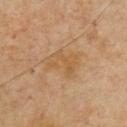Notes:
• follow-up — total-body-photography surveillance lesion; no biopsy
• illumination — cross-polarized illumination
• anatomic site — the chest
• automated lesion analysis — an area of roughly 8.5 mm², a shape eccentricity near 0.7, and a shape-asymmetry score of about 0.35 (0 = symmetric); a mean CIELAB color near L≈44 a*≈15 b*≈31 and a lesion–skin lightness drop of about 5; internal color variation of about 2 on a 0–10 scale and peripheral color asymmetry of about 1; a nevus-likeness score of about 0/100 and lesion-presence confidence of about 100/100
• subject — male, aged around 65
• image source — 15 mm crop, total-body photography